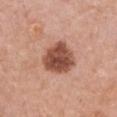This lesion was catalogued during total-body skin photography and was not selected for biopsy.
Cropped from a total-body skin-imaging series; the visible field is about 15 mm.
A female patient aged approximately 60.
The lesion-visualizer software estimated a lesion area of about 13 mm². It also reported a lesion-to-skin contrast of about 11 (normalized; higher = more distinct). The software also gave an automated nevus-likeness rating near 25 out of 100.
The tile uses white-light illumination.
The lesion is located on the front of the torso.
The lesion's longest dimension is about 4 mm.